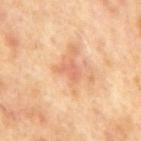Notes:
* notes — catalogued during a skin exam; not biopsied
* imaging modality — 15 mm crop, total-body photography
* illumination — cross-polarized illumination
* lesion diameter — ~3 mm (longest diameter)
* body site — the mid back
* image-analysis metrics — an area of roughly 3.5 mm², an outline eccentricity of about 0.8 (0 = round, 1 = elongated), and a symmetry-axis asymmetry near 0.45; a normalized border contrast of about 5; a border-irregularity index near 4.5/10, internal color variation of about 1 on a 0–10 scale, and peripheral color asymmetry of about 0
* subject — male, aged 63 to 67